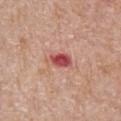Assessment:
Part of a total-body skin-imaging series; this lesion was reviewed on a skin check and was not flagged for biopsy.
Background:
A lesion tile, about 15 mm wide, cut from a 3D total-body photograph. The subject is a male aged 68 to 72. This is a white-light tile. The lesion's longest dimension is about 2.5 mm. From the front of the torso. Automated image analysis of the tile measured an area of roughly 4.5 mm², an outline eccentricity of about 0.8 (0 = round, 1 = elongated), and two-axis asymmetry of about 0.25. It also reported border irregularity of about 2 on a 0–10 scale, internal color variation of about 4.5 on a 0–10 scale, and a peripheral color-asymmetry measure near 1.5.